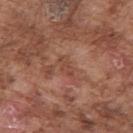biopsy_status: not biopsied; imaged during a skin examination
automated_metrics:
  cielab_L: 46
  cielab_a: 23
  cielab_b: 29
  vs_skin_darker_L: 7.0
  vs_skin_contrast_norm: 5.5
  lesion_detection_confidence_0_100: 90
site: right upper arm
image:
  source: total-body photography crop
  field_of_view_mm: 15
patient:
  sex: male
  age_approx: 75
lesion_size:
  long_diameter_mm_approx: 3.0
lighting: white-light